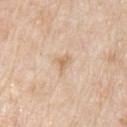Captured during whole-body skin photography for melanoma surveillance; the lesion was not biopsied. Longest diameter approximately 2.5 mm. A 15 mm close-up extracted from a 3D total-body photography capture. The tile uses white-light illumination. A male patient in their 80s. On the arm.